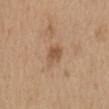biopsy status = total-body-photography surveillance lesion; no biopsy
lighting = white-light
image source = ~15 mm tile from a whole-body skin photo
site = the mid back
subject = male, aged around 70